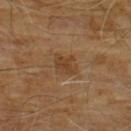Captured during whole-body skin photography for melanoma surveillance; the lesion was not biopsied. Longest diameter approximately 3 mm. This is a cross-polarized tile. A 15 mm crop from a total-body photograph taken for skin-cancer surveillance. Automated tile analysis of the lesion measured a lesion area of about 6 mm², a shape eccentricity near 0.65, and a symmetry-axis asymmetry near 0.3. A male patient, aged approximately 60. The lesion is on the chest.